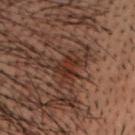Q: Was this lesion biopsied?
A: catalogued during a skin exam; not biopsied
Q: Automated lesion metrics?
A: a normalized border contrast of about 7.5; border irregularity of about 4 on a 0–10 scale, internal color variation of about 3.5 on a 0–10 scale, and radial color variation of about 1.5; a classifier nevus-likeness of about 0/100
Q: Patient demographics?
A: male, aged 48–52
Q: Where on the body is the lesion?
A: the head or neck
Q: What lighting was used for the tile?
A: cross-polarized illumination
Q: What is the imaging modality?
A: ~15 mm tile from a whole-body skin photo
Q: Lesion size?
A: ~3 mm (longest diameter)